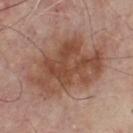follow-up: catalogued during a skin exam; not biopsied
automated lesion analysis: an area of roughly 43 mm² and two-axis asymmetry of about 0.25; a lesion color around L≈49 a*≈21 b*≈28 in CIELAB, about 11 CIELAB-L* units darker than the surrounding skin, and a lesion-to-skin contrast of about 8.5 (normalized; higher = more distinct); border irregularity of about 4.5 on a 0–10 scale and peripheral color asymmetry of about 2
subject: male, about 80 years old
illumination: white-light
body site: the front of the torso
image source: ~15 mm crop, total-body skin-cancer survey
lesion size: ~10 mm (longest diameter)